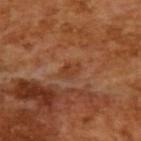Clinical impression: The lesion was tiled from a total-body skin photograph and was not biopsied. Context: Measured at roughly 2.5 mm in maximum diameter. Cropped from a total-body skin-imaging series; the visible field is about 15 mm. Imaged with cross-polarized lighting. A male subject aged 63–67.